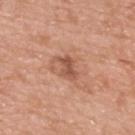Assessment: The lesion was tiled from a total-body skin photograph and was not biopsied. Context: Cropped from a total-body skin-imaging series; the visible field is about 15 mm. Located on the upper back. A male subject aged around 50.